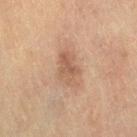Context:
A female patient, approximately 55 years of age. The lesion is on the right thigh. A lesion tile, about 15 mm wide, cut from a 3D total-body photograph.